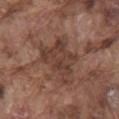Assessment: Part of a total-body skin-imaging series; this lesion was reviewed on a skin check and was not flagged for biopsy. Context: A male subject, aged around 75. The lesion is located on the mid back. Captured under white-light illumination. Cropped from a whole-body photographic skin survey; the tile spans about 15 mm. The total-body-photography lesion software estimated a shape eccentricity near 0.7 and two-axis asymmetry of about 0.35. And it measured a lesion color around L≈39 a*≈19 b*≈25 in CIELAB and roughly 8 lightness units darker than nearby skin. The analysis additionally found a border-irregularity rating of about 5/10 and internal color variation of about 3.5 on a 0–10 scale. The analysis additionally found a nevus-likeness score of about 0/100 and a lesion-detection confidence of about 95/100.